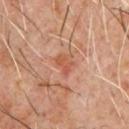  biopsy_status: not biopsied; imaged during a skin examination
  site: chest
  image:
    source: total-body photography crop
    field_of_view_mm: 15
  lesion_size:
    long_diameter_mm_approx: 2.5
  patient:
    sex: male
    age_approx: 60
  automated_metrics:
    area_mm2_approx: 3.0
    eccentricity: 0.8
    shape_asymmetry: 0.35
  lighting: cross-polarized